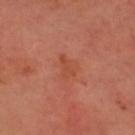Impression:
This lesion was catalogued during total-body skin photography and was not selected for biopsy.
Clinical summary:
Longest diameter approximately 3 mm. A lesion tile, about 15 mm wide, cut from a 3D total-body photograph. A male subject, aged around 55. Imaged with cross-polarized lighting. On the head or neck.